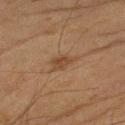site — the right upper arm | imaging modality — ~15 mm tile from a whole-body skin photo | subject — male, in their mid- to late 40s.